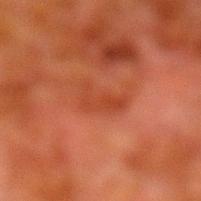Notes:
- workup: total-body-photography surveillance lesion; no biopsy
- patient: male, aged around 80
- anatomic site: the left lower leg
- illumination: cross-polarized illumination
- diameter: ~3.5 mm (longest diameter)
- image source: total-body-photography crop, ~15 mm field of view